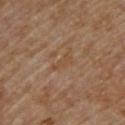{"patient": {"sex": "male", "age_approx": "85+"}, "automated_metrics": {"area_mm2_approx": 2.5, "shape_asymmetry": 0.45, "border_irregularity_0_10": 5.0, "color_variation_0_10": 0.0, "peripheral_color_asymmetry": 0.0, "nevus_likeness_0_100": 0, "lesion_detection_confidence_0_100": 85}, "image": {"source": "total-body photography crop", "field_of_view_mm": 15}, "site": "upper back", "lesion_size": {"long_diameter_mm_approx": 2.5}}